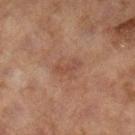site: the right lower leg | lighting: cross-polarized illumination | patient: female, aged 58–62 | lesion diameter: ≈2.5 mm | image: ~15 mm tile from a whole-body skin photo.